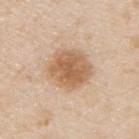Imaged during a routine full-body skin examination; the lesion was not biopsied and no histopathology is available.
Located on the upper back.
Cropped from a whole-body photographic skin survey; the tile spans about 15 mm.
A male subject in their mid-40s.